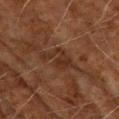Imaged during a routine full-body skin examination; the lesion was not biopsied and no histopathology is available.
The lesion is on the right upper arm.
The recorded lesion diameter is about 5 mm.
The patient is a male approximately 70 years of age.
An algorithmic analysis of the crop reported a border-irregularity index near 8.5/10 and a within-lesion color-variation index near 2.5/10. The analysis additionally found an automated nevus-likeness rating near 0 out of 100 and a lesion-detection confidence of about 85/100.
The tile uses cross-polarized illumination.
A lesion tile, about 15 mm wide, cut from a 3D total-body photograph.